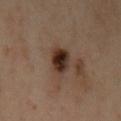This lesion was catalogued during total-body skin photography and was not selected for biopsy. A lesion tile, about 15 mm wide, cut from a 3D total-body photograph. This is a cross-polarized tile. The lesion is on the left upper arm. The lesion-visualizer software estimated a normalized border contrast of about 13. It also reported border irregularity of about 1.5 on a 0–10 scale, a within-lesion color-variation index near 7.5/10, and peripheral color asymmetry of about 2. The patient is a female aged 38 to 42. Measured at roughly 3 mm in maximum diameter.